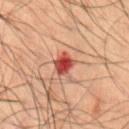workup — total-body-photography surveillance lesion; no biopsy | subject — male, aged approximately 50 | imaging modality — ~15 mm tile from a whole-body skin photo | body site — the abdomen.